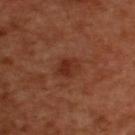follow-up: total-body-photography surveillance lesion; no biopsy | TBP lesion metrics: an area of roughly 5 mm² and an eccentricity of roughly 0.75; a lesion color around L≈31 a*≈26 b*≈30 in CIELAB, a lesion–skin lightness drop of about 7, and a normalized lesion–skin contrast near 7; internal color variation of about 3 on a 0–10 scale; a classifier nevus-likeness of about 25/100 and a detector confidence of about 100 out of 100 that the crop contains a lesion | patient: male, in their 50s | illumination: cross-polarized | size: ≈3 mm | image: ~15 mm tile from a whole-body skin photo | body site: the upper back.